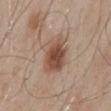biopsy_status: not biopsied; imaged during a skin examination
patient:
  sex: male
  age_approx: 55
lighting: white-light
site: mid back
lesion_size:
  long_diameter_mm_approx: 4.5
image:
  source: total-body photography crop
  field_of_view_mm: 15